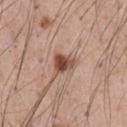Findings:
- notes: catalogued during a skin exam; not biopsied
- acquisition: ~15 mm crop, total-body skin-cancer survey
- location: the chest
- subject: male, in their mid- to late 50s
- lighting: white-light illumination